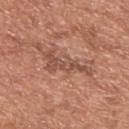A 15 mm close-up extracted from a 3D total-body photography capture. Automated image analysis of the tile measured a footprint of about 8.5 mm², an eccentricity of roughly 0.9, and a shape-asymmetry score of about 0.5 (0 = symmetric). The analysis additionally found a nevus-likeness score of about 0/100 and lesion-presence confidence of about 60/100. Captured under white-light illumination. The recorded lesion diameter is about 6 mm. Located on the upper back. A male patient, roughly 65 years of age.